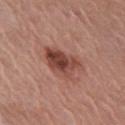This lesion was catalogued during total-body skin photography and was not selected for biopsy.
Located on the right upper arm.
The patient is a female aged 58 to 62.
A 15 mm close-up tile from a total-body photography series done for melanoma screening.
The tile uses white-light illumination.
An algorithmic analysis of the crop reported an area of roughly 12 mm², a shape eccentricity near 0.75, and a symmetry-axis asymmetry near 0.3. It also reported a border-irregularity rating of about 3.5/10, internal color variation of about 7 on a 0–10 scale, and a peripheral color-asymmetry measure near 2.5.
About 5 mm across.